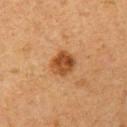{"biopsy_status": "not biopsied; imaged during a skin examination", "automated_metrics": {"area_mm2_approx": 7.5, "eccentricity": 0.55, "shape_asymmetry": 0.2, "border_irregularity_0_10": 1.5, "peripheral_color_asymmetry": 1.5}, "site": "left upper arm", "image": {"source": "total-body photography crop", "field_of_view_mm": 15}, "lesion_size": {"long_diameter_mm_approx": 3.0}, "patient": {"sex": "female", "age_approx": 40}, "lighting": "cross-polarized"}